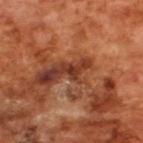diagnosis:
  histopathology: seborrheic keratosis
  malignancy: benign
  taxonomic_path:
    - Benign
    - Benign epidermal proliferations
    - Seborrheic keratosis